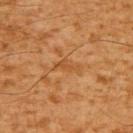Q: Was this lesion biopsied?
A: total-body-photography surveillance lesion; no biopsy
Q: What did automated image analysis measure?
A: border irregularity of about 5.5 on a 0–10 scale and a peripheral color-asymmetry measure near 0; an automated nevus-likeness rating near 0 out of 100 and a detector confidence of about 90 out of 100 that the crop contains a lesion
Q: What is the anatomic site?
A: the upper back
Q: What is the lesion's diameter?
A: about 3 mm
Q: How was this image acquired?
A: total-body-photography crop, ~15 mm field of view
Q: How was the tile lit?
A: cross-polarized illumination
Q: Who is the patient?
A: male, in their 60s